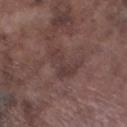Assessment:
Part of a total-body skin-imaging series; this lesion was reviewed on a skin check and was not flagged for biopsy.
Acquisition and patient details:
A close-up tile cropped from a whole-body skin photograph, about 15 mm across. The total-body-photography lesion software estimated border irregularity of about 6.5 on a 0–10 scale, internal color variation of about 2.5 on a 0–10 scale, and a peripheral color-asymmetry measure near 1. And it measured a classifier nevus-likeness of about 0/100 and lesion-presence confidence of about 65/100. This is a white-light tile. On the left lower leg. The recorded lesion diameter is about 5 mm. A male patient roughly 75 years of age.